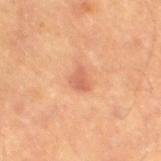workup: imaged on a skin check; not biopsied | image-analysis metrics: an area of roughly 3.5 mm², an eccentricity of roughly 0.7, and a shape-asymmetry score of about 0.4 (0 = symmetric); border irregularity of about 3.5 on a 0–10 scale, a within-lesion color-variation index near 1.5/10, and a peripheral color-asymmetry measure near 0.5 | size: about 2.5 mm | site: the left thigh | image source: total-body-photography crop, ~15 mm field of view | patient: male, in their mid-80s or older | illumination: cross-polarized.